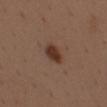Q: Was a biopsy performed?
A: no biopsy performed (imaged during a skin exam)
Q: What are the patient's age and sex?
A: male, aged around 40
Q: How large is the lesion?
A: ~3.5 mm (longest diameter)
Q: What did automated image analysis measure?
A: an area of roughly 5.5 mm², an outline eccentricity of about 0.8 (0 = round, 1 = elongated), and a symmetry-axis asymmetry near 0.2; a border-irregularity index near 2/10 and internal color variation of about 3 on a 0–10 scale
Q: What kind of image is this?
A: 15 mm crop, total-body photography
Q: What is the anatomic site?
A: the mid back